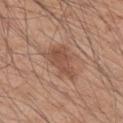Notes:
– follow-up: total-body-photography surveillance lesion; no biopsy
– patient: male, aged approximately 45
– image source: total-body-photography crop, ~15 mm field of view
– lesion size: ≈5 mm
– site: the right upper arm
– tile lighting: white-light illumination